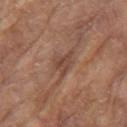Findings:
- patient: male, about 80 years old
- site: the left upper arm
- imaging modality: ~15 mm tile from a whole-body skin photo
- lesion diameter: about 3 mm
- TBP lesion metrics: a border-irregularity rating of about 4/10, internal color variation of about 3.5 on a 0–10 scale, and peripheral color asymmetry of about 1.5; a classifier nevus-likeness of about 0/100 and lesion-presence confidence of about 70/100